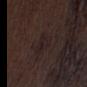Q: Is there a histopathology result?
A: imaged on a skin check; not biopsied
Q: What did automated image analysis measure?
A: a color-variation rating of about 3/10 and radial color variation of about 1
Q: What kind of image is this?
A: ~15 mm crop, total-body skin-cancer survey
Q: What is the anatomic site?
A: the right thigh
Q: How large is the lesion?
A: about 4 mm
Q: Patient demographics?
A: male, approximately 70 years of age
Q: Illumination type?
A: white-light illumination